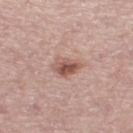This lesion was catalogued during total-body skin photography and was not selected for biopsy.
Automated tile analysis of the lesion measured an area of roughly 5.5 mm² and a symmetry-axis asymmetry near 0.25. It also reported border irregularity of about 2 on a 0–10 scale and internal color variation of about 7 on a 0–10 scale.
A female patient aged approximately 50.
The lesion is located on the left thigh.
A 15 mm crop from a total-body photograph taken for skin-cancer surveillance.
Approximately 3 mm at its widest.